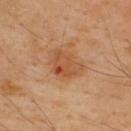This lesion was catalogued during total-body skin photography and was not selected for biopsy.
The lesion is on the upper back.
The subject is a male aged approximately 50.
Cropped from a whole-body photographic skin survey; the tile spans about 15 mm.
The tile uses cross-polarized illumination.